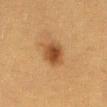The lesion was tiled from a total-body skin photograph and was not biopsied. The lesion-visualizer software estimated an average lesion color of about L≈39 a*≈19 b*≈32 (CIELAB), roughly 11 lightness units darker than nearby skin, and a normalized lesion–skin contrast near 9. The software also gave an automated nevus-likeness rating near 100 out of 100 and a lesion-detection confidence of about 100/100. This is a cross-polarized tile. A female patient aged approximately 40. From the abdomen. A 15 mm crop from a total-body photograph taken for skin-cancer surveillance.